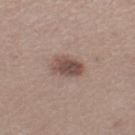Clinical impression:
Captured during whole-body skin photography for melanoma surveillance; the lesion was not biopsied.
Image and clinical context:
The subject is a female about 45 years old. From the left thigh. A lesion tile, about 15 mm wide, cut from a 3D total-body photograph.